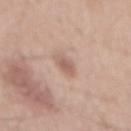An algorithmic analysis of the crop reported an area of roughly 4 mm², a shape eccentricity near 0.75, and a symmetry-axis asymmetry near 0.25. The analysis additionally found a normalized border contrast of about 6. And it measured a border-irregularity index near 2.5/10, a within-lesion color-variation index near 1.5/10, and peripheral color asymmetry of about 0.5. The subject is a male aged 53–57. A 15 mm crop from a total-body photograph taken for skin-cancer surveillance. Measured at roughly 2.5 mm in maximum diameter. Imaged with white-light lighting. The lesion is on the mid back.